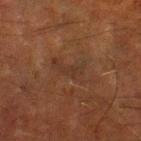The lesion was tiled from a total-body skin photograph and was not biopsied. A region of skin cropped from a whole-body photographic capture, roughly 15 mm wide. Longest diameter approximately 4.5 mm. The subject is a male aged approximately 65. On the right lower leg. The total-body-photography lesion software estimated a footprint of about 6.5 mm², a shape eccentricity near 0.9, and two-axis asymmetry of about 0.55. It also reported about 4 CIELAB-L* units darker than the surrounding skin and a lesion-to-skin contrast of about 4.5 (normalized; higher = more distinct). It also reported border irregularity of about 6.5 on a 0–10 scale and a within-lesion color-variation index near 1.5/10.